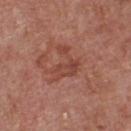Assessment:
The lesion was tiled from a total-body skin photograph and was not biopsied.
Clinical summary:
A region of skin cropped from a whole-body photographic capture, roughly 15 mm wide. The tile uses white-light illumination. A male subject in their mid-50s. From the chest.